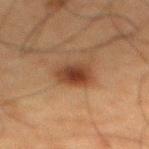The lesion was tiled from a total-body skin photograph and was not biopsied. Located on the mid back. A male patient, aged 58 to 62. A 15 mm close-up extracted from a 3D total-body photography capture. Captured under cross-polarized illumination. Approximately 3.5 mm at its widest.